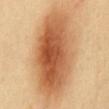notes = catalogued during a skin exam; not biopsied
anatomic site = the mid back
image source = ~15 mm crop, total-body skin-cancer survey
illumination = cross-polarized
patient = female, in their 40s